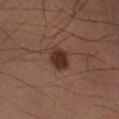Approximately 3 mm at its widest. A male patient approximately 40 years of age. From the right lower leg. This is a cross-polarized tile. Cropped from a total-body skin-imaging series; the visible field is about 15 mm.